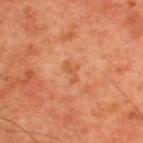Q: Was this lesion biopsied?
A: total-body-photography surveillance lesion; no biopsy
Q: What is the lesion's diameter?
A: ~2.5 mm (longest diameter)
Q: Patient demographics?
A: male, about 60 years old
Q: Lesion location?
A: the upper back
Q: What kind of image is this?
A: ~15 mm crop, total-body skin-cancer survey